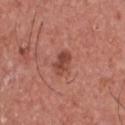Q: Was this lesion biopsied?
A: imaged on a skin check; not biopsied
Q: How was the tile lit?
A: white-light illumination
Q: Lesion location?
A: the upper back
Q: What kind of image is this?
A: 15 mm crop, total-body photography
Q: What are the patient's age and sex?
A: male, in their mid-40s
Q: What is the lesion's diameter?
A: about 3 mm